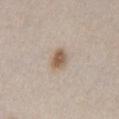The subject is a female approximately 65 years of age. A 15 mm close-up tile from a total-body photography series done for melanoma screening. The tile uses white-light illumination. From the chest. Automated image analysis of the tile measured a lesion-detection confidence of about 100/100. About 2.5 mm across.